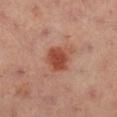subject: female, aged 33–37; illumination: cross-polarized; image: ~15 mm tile from a whole-body skin photo; body site: the right lower leg; lesion size: ≈3.5 mm.